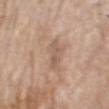Clinical impression: Recorded during total-body skin imaging; not selected for excision or biopsy. Context: This is a white-light tile. A male subject approximately 70 years of age. The lesion is on the head or neck. Cropped from a whole-body photographic skin survey; the tile spans about 15 mm. Automated tile analysis of the lesion measured a shape eccentricity near 0.9 and a shape-asymmetry score of about 0.35 (0 = symmetric). It also reported a border-irregularity index near 5/10, a color-variation rating of about 0/10, and peripheral color asymmetry of about 0. It also reported a classifier nevus-likeness of about 0/100 and lesion-presence confidence of about 100/100.